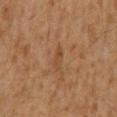This lesion was catalogued during total-body skin photography and was not selected for biopsy. Cropped from a whole-body photographic skin survey; the tile spans about 15 mm. The lesion is located on the mid back. Imaged with cross-polarized lighting. A male patient, roughly 60 years of age. The lesion's longest dimension is about 2.5 mm.